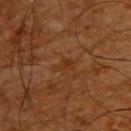<record>
<biopsy_status>not biopsied; imaged during a skin examination</biopsy_status>
<patient>
  <sex>male</sex>
  <age_approx>65</age_approx>
</patient>
<automated_metrics>
  <shape_asymmetry>0.4</shape_asymmetry>
  <border_irregularity_0_10>4.0</border_irregularity_0_10>
  <peripheral_color_asymmetry>0.0</peripheral_color_asymmetry>
  <nevus_likeness_0_100>0</nevus_likeness_0_100>
</automated_metrics>
<site>upper back</site>
<lesion_size>
  <long_diameter_mm_approx>2.5</long_diameter_mm_approx>
</lesion_size>
<image>
  <source>total-body photography crop</source>
  <field_of_view_mm>15</field_of_view_mm>
</image>
</record>